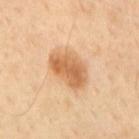Case summary:
• TBP lesion metrics · a color-variation rating of about 4.5/10
• lesion diameter · about 5 mm
• site · the mid back
• patient · male, aged approximately 55
• imaging modality · total-body-photography crop, ~15 mm field of view